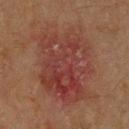Impression:
No biopsy was performed on this lesion — it was imaged during a full skin examination and was not determined to be concerning.
Image and clinical context:
The patient is a male in their 70s. The lesion is located on the back. A 15 mm close-up tile from a total-body photography series done for melanoma screening.